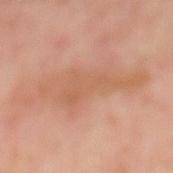biopsy status: no biopsy performed (imaged during a skin exam)
subject: male, aged approximately 50
location: the mid back
imaging modality: total-body-photography crop, ~15 mm field of view
automated metrics: a lesion area of about 20 mm² and an outline eccentricity of about 0.95 (0 = round, 1 = elongated); a border-irregularity rating of about 6.5/10, a color-variation rating of about 2/10, and radial color variation of about 0.5
illumination: cross-polarized illumination
diameter: ≈9 mm